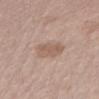| field | value |
|---|---|
| workup | catalogued during a skin exam; not biopsied |
| illumination | white-light |
| image | 15 mm crop, total-body photography |
| body site | the left thigh |
| patient | male, aged approximately 65 |
| lesion diameter | ~4 mm (longest diameter) |
| image-analysis metrics | radial color variation of about 0.5 |